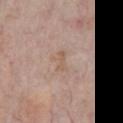notes: no biopsy performed (imaged during a skin exam) | patient: male, aged around 60 | body site: the chest | image source: total-body-photography crop, ~15 mm field of view | image-analysis metrics: an eccentricity of roughly 0.9 and a shape-asymmetry score of about 0.35 (0 = symmetric); an average lesion color of about L≈58 a*≈16 b*≈27 (CIELAB) and a normalized border contrast of about 5; border irregularity of about 4 on a 0–10 scale, a within-lesion color-variation index near 0/10, and peripheral color asymmetry of about 0; an automated nevus-likeness rating near 0 out of 100 and a detector confidence of about 100 out of 100 that the crop contains a lesion | tile lighting: white-light.